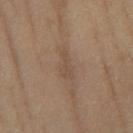Assessment: Recorded during total-body skin imaging; not selected for excision or biopsy. Clinical summary: An algorithmic analysis of the crop reported an area of roughly 4 mm², an eccentricity of roughly 0.9, and two-axis asymmetry of about 0.5. It also reported an average lesion color of about L≈49 a*≈15 b*≈28 (CIELAB) and a lesion-to-skin contrast of about 4.5 (normalized; higher = more distinct). And it measured an automated nevus-likeness rating near 0 out of 100 and a lesion-detection confidence of about 85/100. The lesion is located on the right lower leg. A region of skin cropped from a whole-body photographic capture, roughly 15 mm wide. A female subject aged approximately 70. Captured under cross-polarized illumination. Measured at roughly 3.5 mm in maximum diameter.